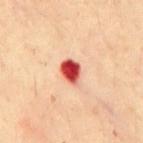{
  "biopsy_status": "not biopsied; imaged during a skin examination",
  "lesion_size": {
    "long_diameter_mm_approx": 3.0
  },
  "lighting": "cross-polarized",
  "site": "mid back",
  "image": {
    "source": "total-body photography crop",
    "field_of_view_mm": 15
  },
  "automated_metrics": {
    "vs_skin_darker_L": 24.0
  },
  "patient": {
    "sex": "male",
    "age_approx": 30
  }
}